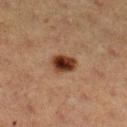Clinical impression:
This lesion was catalogued during total-body skin photography and was not selected for biopsy.
Acquisition and patient details:
The lesion's longest dimension is about 3 mm. A close-up tile cropped from a whole-body skin photograph, about 15 mm across. From the right lower leg. The lesion-visualizer software estimated a lesion color around L≈30 a*≈20 b*≈27 in CIELAB, a lesion–skin lightness drop of about 15, and a normalized border contrast of about 14. It also reported a within-lesion color-variation index near 5.5/10 and a peripheral color-asymmetry measure near 1.5. The subject is a female aged around 40. Imaged with cross-polarized lighting.